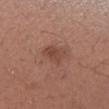  biopsy_status: not biopsied; imaged during a skin examination
  image:
    source: total-body photography crop
    field_of_view_mm: 15
  lesion_size:
    long_diameter_mm_approx: 3.5
  site: right forearm
  automated_metrics:
    cielab_L: 45
    cielab_a: 22
    cielab_b: 27
    vs_skin_darker_L: 8.0
    vs_skin_contrast_norm: 6.0
  patient:
    sex: female
    age_approx: 25